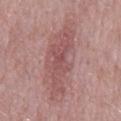Case summary:
– biopsy status: imaged on a skin check; not biopsied
– image-analysis metrics: a footprint of about 19 mm², an eccentricity of roughly 0.95, and a symmetry-axis asymmetry near 0.35; a mean CIELAB color near L≈52 a*≈24 b*≈20 and a normalized lesion–skin contrast near 6.5; a nevus-likeness score of about 0/100 and a lesion-detection confidence of about 75/100
– patient: male, approximately 65 years of age
– diameter: ~8.5 mm (longest diameter)
– image: ~15 mm tile from a whole-body skin photo
– illumination: white-light illumination
– location: the mid back